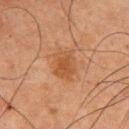Clinical summary: On the front of the torso. The patient is a male roughly 75 years of age. Cropped from a total-body skin-imaging series; the visible field is about 15 mm. Imaged with cross-polarized lighting. The lesion-visualizer software estimated a lesion area of about 9.5 mm², a shape eccentricity near 0.65, and a shape-asymmetry score of about 0.2 (0 = symmetric). The software also gave border irregularity of about 2.5 on a 0–10 scale. The analysis additionally found a nevus-likeness score of about 45/100 and lesion-presence confidence of about 100/100. Measured at roughly 4 mm in maximum diameter.